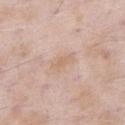The lesion was tiled from a total-body skin photograph and was not biopsied.
A 15 mm close-up tile from a total-body photography series done for melanoma screening.
A male subject, aged around 55.
Imaged with white-light lighting.
Located on the right thigh.